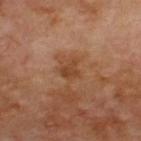Part of a total-body skin-imaging series; this lesion was reviewed on a skin check and was not flagged for biopsy. The lesion is located on the upper back. Captured under cross-polarized illumination. An algorithmic analysis of the crop reported a footprint of about 4.5 mm², an outline eccentricity of about 0.5 (0 = round, 1 = elongated), and a shape-asymmetry score of about 0.4 (0 = symmetric). The analysis additionally found a mean CIELAB color near L≈42 a*≈22 b*≈33, a lesion–skin lightness drop of about 7, and a normalized lesion–skin contrast near 6.5. The analysis additionally found a border-irregularity index near 3.5/10, a color-variation rating of about 2/10, and peripheral color asymmetry of about 0.5. It also reported a lesion-detection confidence of about 100/100. A roughly 15 mm field-of-view crop from a total-body skin photograph. The subject is a male about 70 years old.